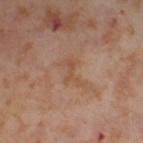Q: Was this lesion biopsied?
A: catalogued during a skin exam; not biopsied
Q: How was this image acquired?
A: ~15 mm crop, total-body skin-cancer survey
Q: Illumination type?
A: cross-polarized
Q: Where on the body is the lesion?
A: the right thigh
Q: How large is the lesion?
A: ≈3.5 mm
Q: Patient demographics?
A: female, roughly 55 years of age